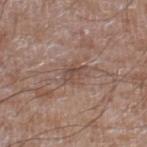<lesion>
  <biopsy_status>not biopsied; imaged during a skin examination</biopsy_status>
  <site>right lower leg</site>
  <patient>
    <sex>male</sex>
    <age_approx>60</age_approx>
  </patient>
  <automated_metrics>
    <cielab_L>47</cielab_L>
    <cielab_a>17</cielab_a>
    <cielab_b>24</cielab_b>
    <vs_skin_contrast_norm>6.0</vs_skin_contrast_norm>
    <border_irregularity_0_10>3.0</border_irregularity_0_10>
    <color_variation_0_10>3.5</color_variation_0_10>
    <peripheral_color_asymmetry>1.5</peripheral_color_asymmetry>
    <lesion_detection_confidence_0_100>95</lesion_detection_confidence_0_100>
  </automated_metrics>
  <image>
    <source>total-body photography crop</source>
    <field_of_view_mm>15</field_of_view_mm>
  </image>
  <lesion_size>
    <long_diameter_mm_approx>2.5</long_diameter_mm_approx>
  </lesion_size>
</lesion>